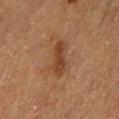Assessment:
Recorded during total-body skin imaging; not selected for excision or biopsy.
Clinical summary:
The lesion-visualizer software estimated a border-irregularity rating of about 3.5/10 and a color-variation rating of about 2/10. The tile uses cross-polarized illumination. The patient is a male aged 73 to 77. On the left thigh. A roughly 15 mm field-of-view crop from a total-body skin photograph.